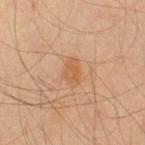Background:
The recorded lesion diameter is about 3 mm. A male subject, aged around 45. This image is a 15 mm lesion crop taken from a total-body photograph. The tile uses cross-polarized illumination. The lesion is located on the left upper arm.